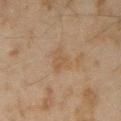biopsy status: no biopsy performed (imaged during a skin exam)
image source: ~15 mm crop, total-body skin-cancer survey
lesion size: ~3 mm (longest diameter)
subject: male, aged 43–47
site: the left forearm
automated metrics: an eccentricity of roughly 0.6 and a shape-asymmetry score of about 0.35 (0 = symmetric); an average lesion color of about L≈43 a*≈13 b*≈28 (CIELAB) and a normalized border contrast of about 5; a border-irregularity index near 3.5/10, a within-lesion color-variation index near 1.5/10, and peripheral color asymmetry of about 0.5; an automated nevus-likeness rating near 0 out of 100 and lesion-presence confidence of about 100/100
illumination: cross-polarized